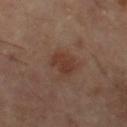  biopsy_status: not biopsied; imaged during a skin examination
  site: left thigh
  image:
    source: total-body photography crop
    field_of_view_mm: 15
  lesion_size:
    long_diameter_mm_approx: 3.5